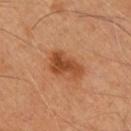No biopsy was performed on this lesion — it was imaged during a full skin examination and was not determined to be concerning. The patient is a male in their mid-50s. On the front of the torso. This image is a 15 mm lesion crop taken from a total-body photograph.